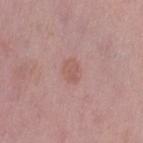- image — total-body-photography crop, ~15 mm field of view
- subject — female, aged around 50
- anatomic site — the left thigh